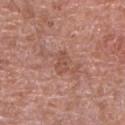Impression: This lesion was catalogued during total-body skin photography and was not selected for biopsy. Clinical summary: The lesion's longest dimension is about 3 mm. This is a white-light tile. Cropped from a whole-body photographic skin survey; the tile spans about 15 mm. The lesion-visualizer software estimated a lesion color around L≈51 a*≈23 b*≈28 in CIELAB, a lesion–skin lightness drop of about 7, and a normalized lesion–skin contrast near 5.5. It also reported border irregularity of about 3 on a 0–10 scale, internal color variation of about 2 on a 0–10 scale, and radial color variation of about 1. Located on the right upper arm. The subject is a male aged 78–82.